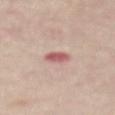The lesion was photographed on a routine skin check and not biopsied; there is no pathology result.
A region of skin cropped from a whole-body photographic capture, roughly 15 mm wide.
The patient is a male in their mid-50s.
Automated tile analysis of the lesion measured an eccentricity of roughly 0.55 and a shape-asymmetry score of about 0.2 (0 = symmetric). The analysis additionally found an average lesion color of about L≈58 a*≈27 b*≈21 (CIELAB), about 13 CIELAB-L* units darker than the surrounding skin, and a lesion-to-skin contrast of about 8.5 (normalized; higher = more distinct). The analysis additionally found an automated nevus-likeness rating near 0 out of 100 and a detector confidence of about 100 out of 100 that the crop contains a lesion.
Captured under white-light illumination.
The lesion is located on the mid back.
Approximately 2.5 mm at its widest.